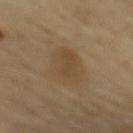Q: Is there a histopathology result?
A: no biopsy performed (imaged during a skin exam)
Q: Illumination type?
A: cross-polarized
Q: What did automated image analysis measure?
A: an area of roughly 14 mm² and two-axis asymmetry of about 0.2
Q: What kind of image is this?
A: ~15 mm tile from a whole-body skin photo
Q: Where on the body is the lesion?
A: the chest
Q: Who is the patient?
A: male, in their mid-60s
Q: What is the lesion's diameter?
A: about 5 mm